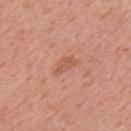Findings:
• notes · no biopsy performed (imaged during a skin exam)
• patient · male, aged 53–57
• image-analysis metrics · a lesion area of about 4 mm² and a shape eccentricity near 0.9; a lesion color around L≈57 a*≈26 b*≈32 in CIELAB, a lesion–skin lightness drop of about 7, and a normalized lesion–skin contrast near 5; a border-irregularity rating of about 4/10, a color-variation rating of about 1.5/10, and a peripheral color-asymmetry measure near 0.5
• image · 15 mm crop, total-body photography
• location · the mid back
• tile lighting · white-light illumination
• size · ~3.5 mm (longest diameter)